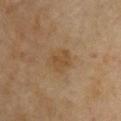Imaged during a routine full-body skin examination; the lesion was not biopsied and no histopathology is available.
The lesion is on the chest.
A 15 mm close-up tile from a total-body photography series done for melanoma screening.
The lesion's longest dimension is about 3 mm.
A female patient, aged 58 to 62.